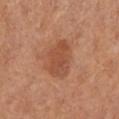Q: Was a biopsy performed?
A: total-body-photography surveillance lesion; no biopsy
Q: Lesion location?
A: the left lower leg
Q: Who is the patient?
A: female, in their mid- to late 60s
Q: Lesion size?
A: ~4 mm (longest diameter)
Q: What is the imaging modality?
A: ~15 mm crop, total-body skin-cancer survey
Q: What did automated image analysis measure?
A: a lesion color around L≈50 a*≈25 b*≈34 in CIELAB and a normalized lesion–skin contrast near 6.5; border irregularity of about 3 on a 0–10 scale and a within-lesion color-variation index near 3.5/10; a classifier nevus-likeness of about 65/100 and a lesion-detection confidence of about 100/100